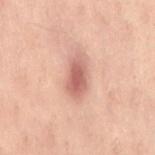Case summary:
- workup · imaged on a skin check; not biopsied
- acquisition · total-body-photography crop, ~15 mm field of view
- patient · female, aged 53 to 57
- diameter · about 4.5 mm
- automated metrics · a mean CIELAB color near L≈51 a*≈21 b*≈23, roughly 11 lightness units darker than nearby skin, and a lesion-to-skin contrast of about 8 (normalized; higher = more distinct); a detector confidence of about 100 out of 100 that the crop contains a lesion
- body site · the lower back